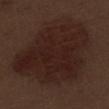Recorded during total-body skin imaging; not selected for excision or biopsy. The lesion is located on the left thigh. The lesion-visualizer software estimated a border-irregularity index near 3.5/10, a color-variation rating of about 3.5/10, and a peripheral color-asymmetry measure near 1. The analysis additionally found a nevus-likeness score of about 0/100. Approximately 11 mm at its widest. Imaged with white-light lighting. The subject is a male aged 68–72. A close-up tile cropped from a whole-body skin photograph, about 15 mm across.